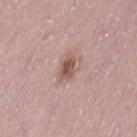Q: Was this lesion biopsied?
A: no biopsy performed (imaged during a skin exam)
Q: What is the anatomic site?
A: the left thigh
Q: What are the patient's age and sex?
A: female, in their 30s
Q: What did automated image analysis measure?
A: an area of roughly 6 mm², an outline eccentricity of about 0.8 (0 = round, 1 = elongated), and a shape-asymmetry score of about 0.25 (0 = symmetric); an automated nevus-likeness rating near 85 out of 100 and a lesion-detection confidence of about 100/100
Q: How was this image acquired?
A: total-body-photography crop, ~15 mm field of view
Q: What lighting was used for the tile?
A: white-light